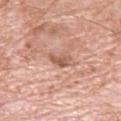{"biopsy_status": "not biopsied; imaged during a skin examination"}